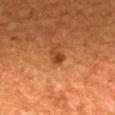Impression:
Recorded during total-body skin imaging; not selected for excision or biopsy.
Clinical summary:
Cropped from a total-body skin-imaging series; the visible field is about 15 mm. A male subject, roughly 45 years of age. About 2 mm across. The lesion is located on the upper back. This is a cross-polarized tile.